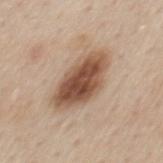The lesion was photographed on a routine skin check and not biopsied; there is no pathology result. A 15 mm close-up tile from a total-body photography series done for melanoma screening. Longest diameter approximately 7 mm. The subject is a male roughly 60 years of age. From the mid back. Captured under white-light illumination.